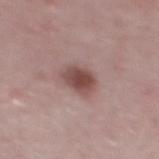Impression: No biopsy was performed on this lesion — it was imaged during a full skin examination and was not determined to be concerning. Context: The recorded lesion diameter is about 3 mm. A male subject, aged 48 to 52. The tile uses white-light illumination. Cropped from a whole-body photographic skin survey; the tile spans about 15 mm. The lesion-visualizer software estimated a lesion color around L≈47 a*≈20 b*≈21 in CIELAB. On the mid back.